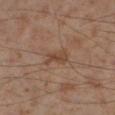Clinical impression: Recorded during total-body skin imaging; not selected for excision or biopsy. Context: The lesion-visualizer software estimated a peripheral color-asymmetry measure near 0.5. The software also gave a classifier nevus-likeness of about 0/100 and a detector confidence of about 100 out of 100 that the crop contains a lesion. This image is a 15 mm lesion crop taken from a total-body photograph. On the left lower leg. This is a cross-polarized tile. A male patient, in their mid-50s.